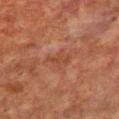{"biopsy_status": "not biopsied; imaged during a skin examination", "lighting": "cross-polarized", "site": "chest", "lesion_size": {"long_diameter_mm_approx": 3.5}, "image": {"source": "total-body photography crop", "field_of_view_mm": 15}, "patient": {"sex": "male", "age_approx": 65}, "automated_metrics": {"border_irregularity_0_10": 4.0, "color_variation_0_10": 1.0, "peripheral_color_asymmetry": 0.5}}